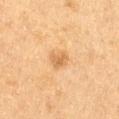Q: Is there a histopathology result?
A: imaged on a skin check; not biopsied
Q: Lesion location?
A: the abdomen
Q: Lesion size?
A: about 2.5 mm
Q: What are the patient's age and sex?
A: female, in their mid- to late 50s
Q: Illumination type?
A: cross-polarized
Q: How was this image acquired?
A: total-body-photography crop, ~15 mm field of view
Q: What did automated image analysis measure?
A: an area of roughly 4.5 mm² and two-axis asymmetry of about 0.2; an average lesion color of about L≈58 a*≈19 b*≈38 (CIELAB), roughly 8 lightness units darker than nearby skin, and a normalized lesion–skin contrast near 6